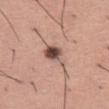<case>
  <biopsy_status>not biopsied; imaged during a skin examination</biopsy_status>
  <site>left thigh</site>
  <lighting>white-light</lighting>
  <lesion_size>
    <long_diameter_mm_approx>3.5</long_diameter_mm_approx>
  </lesion_size>
  <patient>
    <sex>male</sex>
    <age_approx>45</age_approx>
  </patient>
  <image>
    <source>total-body photography crop</source>
    <field_of_view_mm>15</field_of_view_mm>
  </image>
</case>